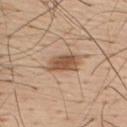Clinical impression:
No biopsy was performed on this lesion — it was imaged during a full skin examination and was not determined to be concerning.
Clinical summary:
A male subject aged 53–57. Captured under white-light illumination. The lesion is located on the upper back. A lesion tile, about 15 mm wide, cut from a 3D total-body photograph. The total-body-photography lesion software estimated a footprint of about 7.5 mm² and an eccentricity of roughly 0.9. The analysis additionally found border irregularity of about 2.5 on a 0–10 scale and internal color variation of about 3.5 on a 0–10 scale. And it measured a nevus-likeness score of about 95/100 and a lesion-detection confidence of about 100/100. Measured at roughly 4.5 mm in maximum diameter.